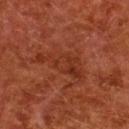workup: imaged on a skin check; not biopsied | subject: female, roughly 50 years of age | image source: ~15 mm crop, total-body skin-cancer survey | diameter: about 6.5 mm | site: the back | TBP lesion metrics: an area of roughly 12 mm², a shape eccentricity near 0.9, and a shape-asymmetry score of about 0.55 (0 = symmetric); an average lesion color of about L≈27 a*≈24 b*≈27 (CIELAB), a lesion–skin lightness drop of about 5, and a lesion-to-skin contrast of about 5.5 (normalized; higher = more distinct); peripheral color asymmetry of about 1 | tile lighting: cross-polarized illumination.